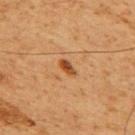Impression:
No biopsy was performed on this lesion — it was imaged during a full skin examination and was not determined to be concerning.
Clinical summary:
Automated tile analysis of the lesion measured an area of roughly 3 mm², an eccentricity of roughly 0.85, and a shape-asymmetry score of about 0.25 (0 = symmetric). And it measured a border-irregularity rating of about 2/10, internal color variation of about 2 on a 0–10 scale, and peripheral color asymmetry of about 0.5. The analysis additionally found a classifier nevus-likeness of about 95/100 and lesion-presence confidence of about 100/100. A 15 mm crop from a total-body photograph taken for skin-cancer surveillance. Imaged with cross-polarized lighting. Located on the back. A male subject, approximately 60 years of age. The lesion's longest dimension is about 2.5 mm.